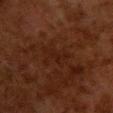On the upper back. The subject is a female roughly 50 years of age. Automated tile analysis of the lesion measured an area of roughly 4.5 mm² and a shape eccentricity near 0.9. It also reported an average lesion color of about L≈16 a*≈18 b*≈21 (CIELAB) and roughly 3 lightness units darker than nearby skin. It also reported a border-irregularity rating of about 7/10 and peripheral color asymmetry of about 0.5. This is a cross-polarized tile. A region of skin cropped from a whole-body photographic capture, roughly 15 mm wide.